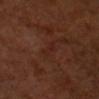Notes:
• notes: no biopsy performed (imaged during a skin exam)
• image-analysis metrics: an outline eccentricity of about 0.95 (0 = round, 1 = elongated) and two-axis asymmetry of about 0.35; an average lesion color of about L≈23 a*≈21 b*≈24 (CIELAB)
• lesion diameter: ~3 mm (longest diameter)
• subject: male, roughly 50 years of age
• body site: the head or neck
• tile lighting: cross-polarized illumination
• acquisition: 15 mm crop, total-body photography